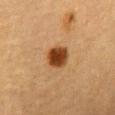follow-up: imaged on a skin check; not biopsied
tile lighting: cross-polarized illumination
anatomic site: the abdomen
diameter: ≈3 mm
imaging modality: 15 mm crop, total-body photography
patient: female, aged 53–57